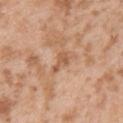<record>
  <biopsy_status>not biopsied; imaged during a skin examination</biopsy_status>
  <automated_metrics>
    <shape_asymmetry>0.5</shape_asymmetry>
    <border_irregularity_0_10>5.5</border_irregularity_0_10>
    <peripheral_color_asymmetry>0.5</peripheral_color_asymmetry>
    <nevus_likeness_0_100>0</nevus_likeness_0_100>
  </automated_metrics>
  <lighting>white-light</lighting>
  <image>
    <source>total-body photography crop</source>
    <field_of_view_mm>15</field_of_view_mm>
  </image>
  <lesion_size>
    <long_diameter_mm_approx>3.0</long_diameter_mm_approx>
  </lesion_size>
  <site>upper back</site>
  <patient>
    <sex>male</sex>
    <age_approx>25</age_approx>
  </patient>
</record>